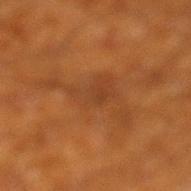Findings:
• workup: catalogued during a skin exam; not biopsied
• location: the left lower leg
• image: ~15 mm crop, total-body skin-cancer survey
• patient: male, in their 60s
• image-analysis metrics: an area of roughly 14 mm², an outline eccentricity of about 0.9 (0 = round, 1 = elongated), and two-axis asymmetry of about 0.65; a border-irregularity rating of about 10/10 and a peripheral color-asymmetry measure near 0.5; a classifier nevus-likeness of about 0/100 and a lesion-detection confidence of about 85/100
• lighting: cross-polarized